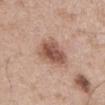Cropped from a total-body skin-imaging series; the visible field is about 15 mm.
Captured under white-light illumination.
Approximately 4.5 mm at its widest.
The lesion is on the front of the torso.
A male patient, aged 63 to 67.